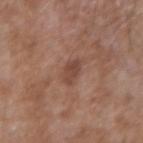Clinical impression:
Captured during whole-body skin photography for melanoma surveillance; the lesion was not biopsied.
Acquisition and patient details:
The lesion's longest dimension is about 2.5 mm. Imaged with white-light lighting. Cropped from a total-body skin-imaging series; the visible field is about 15 mm. The total-body-photography lesion software estimated a shape-asymmetry score of about 0.3 (0 = symmetric). The software also gave a classifier nevus-likeness of about 15/100. Located on the left forearm. A male patient, in their mid- to late 50s.